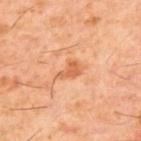<record>
<automated_metrics>
  <cielab_L>58</cielab_L>
  <cielab_a>26</cielab_a>
  <cielab_b>38</cielab_b>
  <vs_skin_darker_L>9.0</vs_skin_darker_L>
  <vs_skin_contrast_norm>6.5</vs_skin_contrast_norm>
  <color_variation_0_10>1.5</color_variation_0_10>
  <peripheral_color_asymmetry>0.5</peripheral_color_asymmetry>
</automated_metrics>
<lighting>cross-polarized</lighting>
<lesion_size>
  <long_diameter_mm_approx>3.0</long_diameter_mm_approx>
</lesion_size>
<site>upper back</site>
<patient>
  <sex>male</sex>
  <age_approx>60</age_approx>
</patient>
<image>
  <source>total-body photography crop</source>
  <field_of_view_mm>15</field_of_view_mm>
</image>
</record>